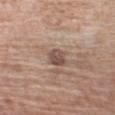Impression: Captured during whole-body skin photography for melanoma surveillance; the lesion was not biopsied. Acquisition and patient details: Approximately 2.5 mm at its widest. Imaged with white-light lighting. The lesion is located on the left upper arm. A region of skin cropped from a whole-body photographic capture, roughly 15 mm wide. The subject is a male aged 58–62. The total-body-photography lesion software estimated a footprint of about 4.5 mm², an outline eccentricity of about 0.6 (0 = round, 1 = elongated), and a shape-asymmetry score of about 0.3 (0 = symmetric). The analysis additionally found a within-lesion color-variation index near 3/10. The analysis additionally found a lesion-detection confidence of about 100/100.